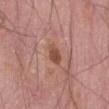Clinical impression: Captured during whole-body skin photography for melanoma surveillance; the lesion was not biopsied. Image and clinical context: The lesion is on the abdomen. Imaged with white-light lighting. Cropped from a whole-body photographic skin survey; the tile spans about 15 mm. Approximately 3 mm at its widest. A male patient, aged around 75.